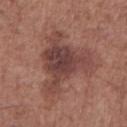workup=no biopsy performed (imaged during a skin exam)
diameter=~8 mm (longest diameter)
subject=male, in their mid-70s
anatomic site=the chest
image source=15 mm crop, total-body photography